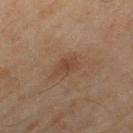The lesion was photographed on a routine skin check and not biopsied; there is no pathology result.
A female subject aged approximately 60.
From the right thigh.
A 15 mm close-up extracted from a 3D total-body photography capture.
Automated tile analysis of the lesion measured a classifier nevus-likeness of about 5/100 and lesion-presence confidence of about 100/100.
Measured at roughly 3 mm in maximum diameter.
Imaged with cross-polarized lighting.